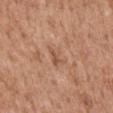lesion_size:
  long_diameter_mm_approx: 2.5
site: mid back
image:
  source: total-body photography crop
  field_of_view_mm: 15
patient:
  sex: male
  age_approx: 60